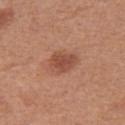The lesion was photographed on a routine skin check and not biopsied; there is no pathology result.
The patient is a female roughly 40 years of age.
Imaged with white-light lighting.
The recorded lesion diameter is about 3.5 mm.
A close-up tile cropped from a whole-body skin photograph, about 15 mm across.
The lesion is on the left thigh.